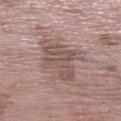The lesion was tiled from a total-body skin photograph and was not biopsied. A roughly 15 mm field-of-view crop from a total-body skin photograph. The lesion is on the left lower leg. A female subject, aged around 70.